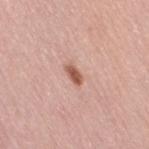<tbp_lesion>
<biopsy_status>not biopsied; imaged during a skin examination</biopsy_status>
<automated_metrics>
  <area_mm2_approx>3.0</area_mm2_approx>
  <shape_asymmetry>0.25</shape_asymmetry>
  <color_variation_0_10>2.5</color_variation_0_10>
  <peripheral_color_asymmetry>1.0</peripheral_color_asymmetry>
</automated_metrics>
<site>right thigh</site>
<lesion_size>
  <long_diameter_mm_approx>2.5</long_diameter_mm_approx>
</lesion_size>
<image>
  <source>total-body photography crop</source>
  <field_of_view_mm>15</field_of_view_mm>
</image>
<patient>
  <sex>female</sex>
  <age_approx>65</age_approx>
</patient>
<lighting>white-light</lighting>
</tbp_lesion>